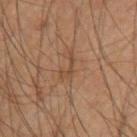* biopsy status: total-body-photography surveillance lesion; no biopsy
* imaging modality: total-body-photography crop, ~15 mm field of view
* patient: male, approximately 60 years of age
* site: the right upper arm
* illumination: cross-polarized illumination